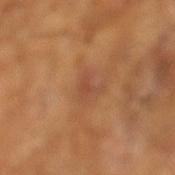Assessment: Captured during whole-body skin photography for melanoma surveillance; the lesion was not biopsied. Background: A male patient, about 65 years old. The tile uses cross-polarized illumination. Measured at roughly 2.5 mm in maximum diameter. A 15 mm close-up extracted from a 3D total-body photography capture.